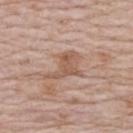• workup — catalogued during a skin exam; not biopsied
• image — total-body-photography crop, ~15 mm field of view
• location — the upper back
• subject — female, aged 63–67